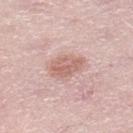{
  "biopsy_status": "not biopsied; imaged during a skin examination",
  "image": {
    "source": "total-body photography crop",
    "field_of_view_mm": 15
  },
  "lighting": "white-light",
  "patient": {
    "sex": "male",
    "age_approx": 50
  },
  "site": "left lower leg"
}